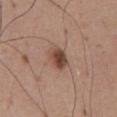<lesion>
<biopsy_status>not biopsied; imaged during a skin examination</biopsy_status>
<automated_metrics>
  <cielab_L>45</cielab_L>
  <cielab_a>20</cielab_a>
  <cielab_b>26</cielab_b>
  <vs_skin_darker_L>13.0</vs_skin_darker_L>
  <vs_skin_contrast_norm>9.5</vs_skin_contrast_norm>
  <border_irregularity_0_10>1.5</border_irregularity_0_10>
  <peripheral_color_asymmetry>1.5</peripheral_color_asymmetry>
  <nevus_likeness_0_100>95</nevus_likeness_0_100>
  <lesion_detection_confidence_0_100>100</lesion_detection_confidence_0_100>
</automated_metrics>
<lesion_size>
  <long_diameter_mm_approx>3.0</long_diameter_mm_approx>
</lesion_size>
<lighting>white-light</lighting>
<image>
  <source>total-body photography crop</source>
  <field_of_view_mm>15</field_of_view_mm>
</image>
<site>abdomen</site>
<patient>
  <sex>male</sex>
  <age_approx>65</age_approx>
</patient>
</lesion>